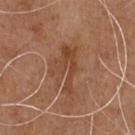Recorded during total-body skin imaging; not selected for excision or biopsy. An algorithmic analysis of the crop reported a lesion color around L≈44 a*≈23 b*≈32 in CIELAB, about 8 CIELAB-L* units darker than the surrounding skin, and a lesion-to-skin contrast of about 6 (normalized; higher = more distinct). It also reported peripheral color asymmetry of about 1. It also reported a nevus-likeness score of about 0/100 and a lesion-detection confidence of about 100/100. A lesion tile, about 15 mm wide, cut from a 3D total-body photograph. The lesion is located on the front of the torso. A male patient in their 70s. Imaged with white-light lighting.